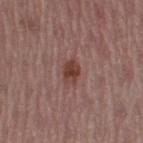notes=no biopsy performed (imaged during a skin exam) | anatomic site=the right thigh | tile lighting=white-light | acquisition=~15 mm tile from a whole-body skin photo | patient=female, in their mid-50s.